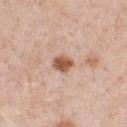Recorded during total-body skin imaging; not selected for excision or biopsy. From the chest. Captured under white-light illumination. Approximately 2.5 mm at its widest. A male subject, roughly 50 years of age. A region of skin cropped from a whole-body photographic capture, roughly 15 mm wide. Automated image analysis of the tile measured an average lesion color of about L≈56 a*≈21 b*≈31 (CIELAB), roughly 15 lightness units darker than nearby skin, and a lesion-to-skin contrast of about 10.5 (normalized; higher = more distinct). The software also gave a nevus-likeness score of about 95/100 and lesion-presence confidence of about 100/100.